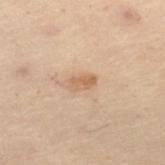tile lighting — cross-polarized
diameter — about 3 mm
patient — female, aged around 50
image — total-body-photography crop, ~15 mm field of view
location — the right lower leg
automated metrics — an outline eccentricity of about 0.85 (0 = round, 1 = elongated) and a symmetry-axis asymmetry near 0.3; an average lesion color of about L≈52 a*≈16 b*≈30 (CIELAB), a lesion–skin lightness drop of about 8, and a normalized border contrast of about 6.5; a lesion-detection confidence of about 100/100